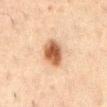<case>
<image>
  <source>total-body photography crop</source>
  <field_of_view_mm>15</field_of_view_mm>
</image>
<patient>
  <sex>male</sex>
  <age_approx>50</age_approx>
</patient>
<site>abdomen</site>
</case>